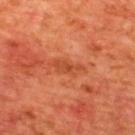follow-up=total-body-photography surveillance lesion; no biopsy | tile lighting=cross-polarized | patient=male, aged 63–67 | size=≈4 mm | automated lesion analysis=an average lesion color of about L≈44 a*≈30 b*≈37 (CIELAB), roughly 7 lightness units darker than nearby skin, and a normalized lesion–skin contrast near 5.5; an automated nevus-likeness rating near 0 out of 100 | acquisition=~15 mm crop, total-body skin-cancer survey | body site=the upper back.